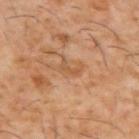<tbp_lesion>
<automated_metrics>
  <eccentricity>0.9</eccentricity>
  <shape_asymmetry>0.5</shape_asymmetry>
</automated_metrics>
<image>
  <source>total-body photography crop</source>
  <field_of_view_mm>15</field_of_view_mm>
</image>
<lesion_size>
  <long_diameter_mm_approx>2.5</long_diameter_mm_approx>
</lesion_size>
<lighting>cross-polarized</lighting>
<patient>
  <sex>male</sex>
  <age_approx>60</age_approx>
</patient>
<site>upper back</site>
</tbp_lesion>